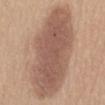Part of a total-body skin-imaging series; this lesion was reviewed on a skin check and was not flagged for biopsy.
Imaged with white-light lighting.
Located on the abdomen.
An algorithmic analysis of the crop reported a lesion color around L≈55 a*≈19 b*≈27 in CIELAB, roughly 12 lightness units darker than nearby skin, and a normalized lesion–skin contrast near 8.5. It also reported a classifier nevus-likeness of about 30/100 and a detector confidence of about 100 out of 100 that the crop contains a lesion.
A male subject roughly 60 years of age.
The lesion's longest dimension is about 14.5 mm.
A 15 mm close-up tile from a total-body photography series done for melanoma screening.